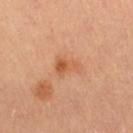Assessment:
Imaged during a routine full-body skin examination; the lesion was not biopsied and no histopathology is available.
Image and clinical context:
A close-up tile cropped from a whole-body skin photograph, about 15 mm across. Imaged with cross-polarized lighting. The recorded lesion diameter is about 3.5 mm. The lesion is located on the right thigh. A female patient, aged 58–62.